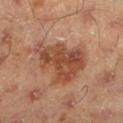Findings:
* notes · no biopsy performed (imaged during a skin exam)
* TBP lesion metrics · a lesion color around L≈47 a*≈23 b*≈32 in CIELAB, roughly 11 lightness units darker than nearby skin, and a normalized lesion–skin contrast near 8.5
* anatomic site · the left lower leg
* patient · male, about 45 years old
* size · about 7 mm
* tile lighting · cross-polarized illumination
* image source · ~15 mm crop, total-body skin-cancer survey